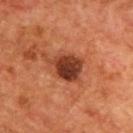<tbp_lesion>
<biopsy_status>not biopsied; imaged during a skin examination</biopsy_status>
<lighting>cross-polarized</lighting>
<patient>
  <sex>male</sex>
  <age_approx>55</age_approx>
</patient>
<image>
  <source>total-body photography crop</source>
  <field_of_view_mm>15</field_of_view_mm>
</image>
<site>chest</site>
<lesion_size>
  <long_diameter_mm_approx>5.5</long_diameter_mm_approx>
</lesion_size>
</tbp_lesion>